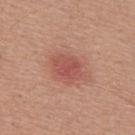This lesion was catalogued during total-body skin photography and was not selected for biopsy.
A 15 mm crop from a total-body photograph taken for skin-cancer surveillance.
The recorded lesion diameter is about 3.5 mm.
An algorithmic analysis of the crop reported an area of roughly 8 mm², an outline eccentricity of about 0.65 (0 = round, 1 = elongated), and a shape-asymmetry score of about 0.2 (0 = symmetric). The software also gave a lesion color around L≈52 a*≈28 b*≈27 in CIELAB, about 9 CIELAB-L* units darker than the surrounding skin, and a normalized lesion–skin contrast near 6. The analysis additionally found a nevus-likeness score of about 5/100 and a lesion-detection confidence of about 100/100.
Located on the mid back.
The patient is a male aged 53–57.
The tile uses white-light illumination.